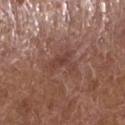| feature | finding |
|---|---|
| biopsy status | imaged on a skin check; not biopsied |
| lighting | white-light illumination |
| subject | male, in their mid- to late 70s |
| lesion size | ~3.5 mm (longest diameter) |
| image source | 15 mm crop, total-body photography |
| location | the left lower leg |